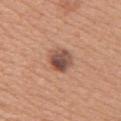Q: Was a biopsy performed?
A: imaged on a skin check; not biopsied
Q: What lighting was used for the tile?
A: white-light illumination
Q: What is the lesion's diameter?
A: about 3 mm
Q: What is the imaging modality?
A: ~15 mm crop, total-body skin-cancer survey
Q: What are the patient's age and sex?
A: female, in their mid-40s
Q: Lesion location?
A: the arm